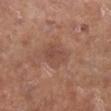{
  "biopsy_status": "not biopsied; imaged during a skin examination",
  "automated_metrics": {
    "area_mm2_approx": 7.5,
    "eccentricity": 0.55,
    "shape_asymmetry": 0.2,
    "border_irregularity_0_10": 2.0,
    "color_variation_0_10": 2.5,
    "nevus_likeness_0_100": 0,
    "lesion_detection_confidence_0_100": 100
  },
  "patient": {
    "sex": "male",
    "age_approx": 70
  },
  "lighting": "white-light",
  "lesion_size": {
    "long_diameter_mm_approx": 3.5
  },
  "image": {
    "source": "total-body photography crop",
    "field_of_view_mm": 15
  },
  "site": "left lower leg"
}